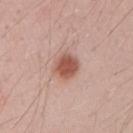notes: imaged on a skin check; not biopsied
imaging modality: total-body-photography crop, ~15 mm field of view
location: the right upper arm
lesion diameter: ≈3 mm
illumination: white-light
subject: male, aged 28–32